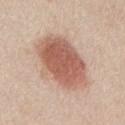On the mid back. This image is a 15 mm lesion crop taken from a total-body photograph. The patient is a male in their 60s.